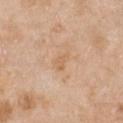Assessment: Recorded during total-body skin imaging; not selected for excision or biopsy. Image and clinical context: Captured under white-light illumination. Automated image analysis of the tile measured border irregularity of about 3.5 on a 0–10 scale and a color-variation rating of about 1.5/10. The analysis additionally found a nevus-likeness score of about 0/100 and a detector confidence of about 100 out of 100 that the crop contains a lesion. A lesion tile, about 15 mm wide, cut from a 3D total-body photograph. On the front of the torso. A female patient approximately 70 years of age. About 2.5 mm across.